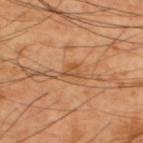Notes:
* workup: imaged on a skin check; not biopsied
* subject: male, aged approximately 60
* location: the upper back
* imaging modality: ~15 mm tile from a whole-body skin photo